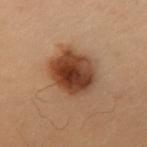Recorded during total-body skin imaging; not selected for excision or biopsy. This is a cross-polarized tile. From the chest. A lesion tile, about 15 mm wide, cut from a 3D total-body photograph. The patient is a female about 50 years old.